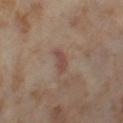Q: Illumination type?
A: cross-polarized
Q: Lesion size?
A: about 2.5 mm
Q: Where on the body is the lesion?
A: the left thigh
Q: What are the patient's age and sex?
A: female, in their mid- to late 50s
Q: How was this image acquired?
A: total-body-photography crop, ~15 mm field of view